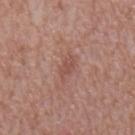No biopsy was performed on this lesion — it was imaged during a full skin examination and was not determined to be concerning.
About 3 mm across.
A roughly 15 mm field-of-view crop from a total-body skin photograph.
Automated image analysis of the tile measured a footprint of about 3.5 mm², a shape eccentricity near 0.8, and two-axis asymmetry of about 0.25. It also reported a lesion–skin lightness drop of about 7 and a normalized lesion–skin contrast near 5.5.
Located on the mid back.
A male patient aged around 65.
This is a white-light tile.